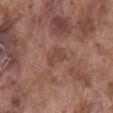biopsy_status: not biopsied; imaged during a skin examination
image:
  source: total-body photography crop
  field_of_view_mm: 15
automated_metrics:
  cielab_L: 44
  cielab_a: 20
  cielab_b: 25
  vs_skin_contrast_norm: 5.5
  border_irregularity_0_10: 3.0
  color_variation_0_10: 1.5
  nevus_likeness_0_100: 0
  lesion_detection_confidence_0_100: 100
site: abdomen
lighting: white-light
patient:
  sex: male
  age_approx: 75